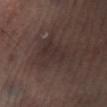Imaged during a routine full-body skin examination; the lesion was not biopsied and no histopathology is available.
An algorithmic analysis of the crop reported a mean CIELAB color near L≈30 a*≈13 b*≈16, about 5 CIELAB-L* units darker than the surrounding skin, and a normalized border contrast of about 5.5. It also reported an automated nevus-likeness rating near 0 out of 100 and a lesion-detection confidence of about 90/100.
Captured under cross-polarized illumination.
Approximately 5 mm at its widest.
The lesion is located on the right lower leg.
This image is a 15 mm lesion crop taken from a total-body photograph.